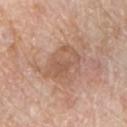Imaged during a routine full-body skin examination; the lesion was not biopsied and no histopathology is available. Captured under white-light illumination. A 15 mm crop from a total-body photograph taken for skin-cancer surveillance. Longest diameter approximately 5 mm. The lesion is located on the chest. Automated image analysis of the tile measured a mean CIELAB color near L≈57 a*≈20 b*≈30 and a lesion–skin lightness drop of about 8. The software also gave a border-irregularity index near 4/10, a color-variation rating of about 4/10, and radial color variation of about 1.5. The analysis additionally found an automated nevus-likeness rating near 0 out of 100 and lesion-presence confidence of about 100/100. The patient is a male aged 58 to 62.